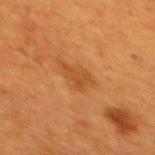Clinical impression:
This lesion was catalogued during total-body skin photography and was not selected for biopsy.
Clinical summary:
This image is a 15 mm lesion crop taken from a total-body photograph. A female patient, aged around 40. On the upper back. The tile uses cross-polarized illumination.